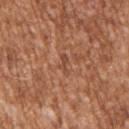Clinical impression: The lesion was photographed on a routine skin check and not biopsied; there is no pathology result. Image and clinical context: Located on the arm. Cropped from a total-body skin-imaging series; the visible field is about 15 mm. The subject is a male aged approximately 45.